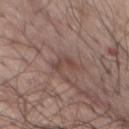| field | value |
|---|---|
| notes | imaged on a skin check; not biopsied |
| lighting | white-light illumination |
| patient | male, approximately 75 years of age |
| diameter | ≈2.5 mm |
| location | the chest |
| imaging modality | ~15 mm tile from a whole-body skin photo |